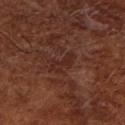| field | value |
|---|---|
| biopsy status | catalogued during a skin exam; not biopsied |
| acquisition | 15 mm crop, total-body photography |
| anatomic site | the right lower leg |
| subject | male, in their 70s |
| lesion diameter | ≈3.5 mm |
| illumination | cross-polarized |